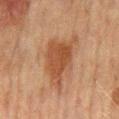A 15 mm crop from a total-body photograph taken for skin-cancer surveillance.
Measured at roughly 6.5 mm in maximum diameter.
A male subject aged 63–67.
Located on the abdomen.